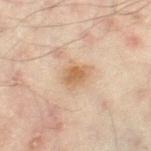biopsy_status: not biopsied; imaged during a skin examination
automated_metrics:
  area_mm2_approx: 6.0
  eccentricity: 0.7
  shape_asymmetry: 0.3
  border_irregularity_0_10: 2.5
  color_variation_0_10: 3.5
  peripheral_color_asymmetry: 1.0
patient:
  sex: male
  age_approx: 45
lighting: cross-polarized
lesion_size:
  long_diameter_mm_approx: 3.5
site: leg
image:
  source: total-body photography crop
  field_of_view_mm: 15